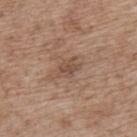Assessment:
Part of a total-body skin-imaging series; this lesion was reviewed on a skin check and was not flagged for biopsy.
Context:
Cropped from a whole-body photographic skin survey; the tile spans about 15 mm. The patient is a male aged approximately 70. The lesion is on the upper back. The lesion-visualizer software estimated an outline eccentricity of about 0.75 (0 = round, 1 = elongated) and a symmetry-axis asymmetry near 0.3. And it measured an average lesion color of about L≈49 a*≈17 b*≈27 (CIELAB) and a normalized lesion–skin contrast near 5.5. And it measured an automated nevus-likeness rating near 0 out of 100 and lesion-presence confidence of about 100/100. The lesion's longest dimension is about 3 mm. This is a white-light tile.